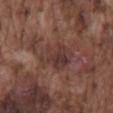Impression: This lesion was catalogued during total-body skin photography and was not selected for biopsy. Context: This is a white-light tile. The lesion is on the mid back. A male patient about 75 years old. A region of skin cropped from a whole-body photographic capture, roughly 15 mm wide.